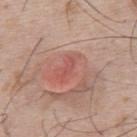The lesion was photographed on a routine skin check and not biopsied; there is no pathology result.
From the upper back.
Captured under white-light illumination.
A male patient in their mid- to late 50s.
The recorded lesion diameter is about 2.5 mm.
A region of skin cropped from a whole-body photographic capture, roughly 15 mm wide.